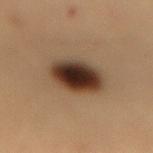Context:
Automated image analysis of the tile measured an average lesion color of about L≈28 a*≈14 b*≈22 (CIELAB), about 16 CIELAB-L* units darker than the surrounding skin, and a normalized border contrast of about 15. The software also gave border irregularity of about 1 on a 0–10 scale and a within-lesion color-variation index near 8/10. The analysis additionally found lesion-presence confidence of about 100/100. The lesion is on the back. Imaged with cross-polarized lighting. A male subject, in their mid- to late 50s. A close-up tile cropped from a whole-body skin photograph, about 15 mm across.